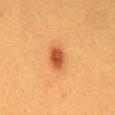notes = total-body-photography surveillance lesion; no biopsy
lighting = cross-polarized illumination
anatomic site = the mid back
subject = female, aged 38–42
diameter = ~3 mm (longest diameter)
acquisition = ~15 mm tile from a whole-body skin photo